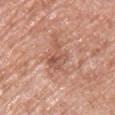  biopsy_status: not biopsied; imaged during a skin examination
  image:
    source: total-body photography crop
    field_of_view_mm: 15
  site: chest
  lighting: white-light
  patient:
    sex: female
    age_approx: 60